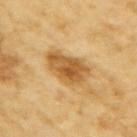• notes: no biopsy performed (imaged during a skin exam)
• patient: female, about 70 years old
• location: the right upper arm
• automated lesion analysis: a footprint of about 13 mm², a shape eccentricity near 0.7, and two-axis asymmetry of about 0.25; a border-irregularity rating of about 2.5/10 and a color-variation rating of about 5/10
• imaging modality: 15 mm crop, total-body photography
• lighting: cross-polarized illumination